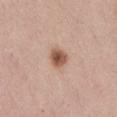This lesion was catalogued during total-body skin photography and was not selected for biopsy. The lesion is located on the lower back. Longest diameter approximately 2.5 mm. A roughly 15 mm field-of-view crop from a total-body skin photograph. Captured under white-light illumination. A female subject in their mid-40s. An algorithmic analysis of the crop reported a mean CIELAB color near L≈55 a*≈21 b*≈29, roughly 14 lightness units darker than nearby skin, and a normalized border contrast of about 9.5. It also reported a peripheral color-asymmetry measure near 1.5.